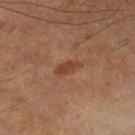workup = no biopsy performed (imaged during a skin exam) | lesion size = ~3.5 mm (longest diameter) | patient = male, aged 63 to 67 | illumination = cross-polarized | anatomic site = the right lower leg | acquisition = total-body-photography crop, ~15 mm field of view.